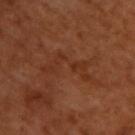notes = total-body-photography surveillance lesion; no biopsy
image = ~15 mm tile from a whole-body skin photo
tile lighting = cross-polarized
body site = the upper back
subject = male, aged 48 to 52